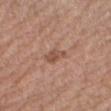Captured during whole-body skin photography for melanoma surveillance; the lesion was not biopsied. From the right upper arm. Captured under white-light illumination. The lesion's longest dimension is about 2.5 mm. Cropped from a total-body skin-imaging series; the visible field is about 15 mm. A male patient, roughly 60 years of age.